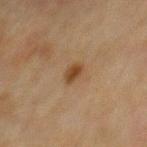workup = imaged on a skin check; not biopsied | diameter = ≈2.5 mm | subject = male, aged around 70 | site = the mid back | imaging modality = total-body-photography crop, ~15 mm field of view.